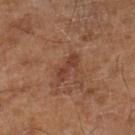The lesion was tiled from a total-body skin photograph and was not biopsied. A lesion tile, about 15 mm wide, cut from a 3D total-body photograph. The total-body-photography lesion software estimated a lesion–skin lightness drop of about 7 and a normalized border contrast of about 6.5. It also reported a classifier nevus-likeness of about 0/100 and lesion-presence confidence of about 100/100. A male subject, aged around 65.